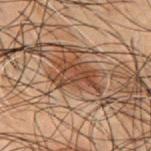{
  "biopsy_status": "not biopsied; imaged during a skin examination",
  "image": {
    "source": "total-body photography crop",
    "field_of_view_mm": 15
  },
  "automated_metrics": {
    "area_mm2_approx": 9.0,
    "border_irregularity_0_10": 6.0,
    "color_variation_0_10": 4.0,
    "peripheral_color_asymmetry": 1.5
  },
  "site": "upper back",
  "lesion_size": {
    "long_diameter_mm_approx": 5.0
  },
  "lighting": "cross-polarized",
  "patient": {
    "sex": "male",
    "age_approx": 50
  }
}